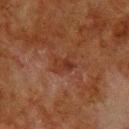Clinical impression: No biopsy was performed on this lesion — it was imaged during a full skin examination and was not determined to be concerning. Clinical summary: A male patient, approximately 80 years of age. The lesion is on the back. The lesion-visualizer software estimated a symmetry-axis asymmetry near 0.3. The analysis additionally found border irregularity of about 3.5 on a 0–10 scale and internal color variation of about 3.5 on a 0–10 scale. Cropped from a whole-body photographic skin survey; the tile spans about 15 mm. This is a cross-polarized tile.